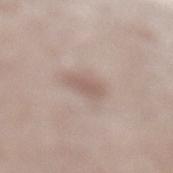Image and clinical context:
From the left lower leg. A region of skin cropped from a whole-body photographic capture, roughly 15 mm wide. A female patient aged around 60.